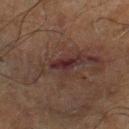Background: A male patient, aged 68 to 72. This is a cross-polarized tile. From the right lower leg. Cropped from a total-body skin-imaging series; the visible field is about 15 mm. Approximately 2.5 mm at its widest. Automated image analysis of the tile measured a color-variation rating of about 1.5/10 and radial color variation of about 0.5. It also reported lesion-presence confidence of about 70/100.